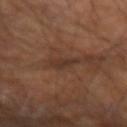follow-up = no biopsy performed (imaged during a skin exam)
patient = male, roughly 60 years of age
site = the left arm
lesion diameter = about 3 mm
illumination = cross-polarized illumination
image = total-body-photography crop, ~15 mm field of view